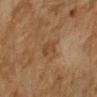Clinical impression: The lesion was photographed on a routine skin check and not biopsied; there is no pathology result. Context: A female subject, aged approximately 55. Cropped from a total-body skin-imaging series; the visible field is about 15 mm. From the right forearm. An algorithmic analysis of the crop reported a lesion-detection confidence of about 100/100.